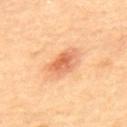Q: Is there a histopathology result?
A: imaged on a skin check; not biopsied